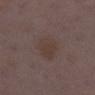image-analysis metrics: a footprint of about 9 mm² and two-axis asymmetry of about 0.2; a normalized lesion–skin contrast near 5.5; a border-irregularity index near 2/10, a color-variation rating of about 2/10, and a peripheral color-asymmetry measure near 0.5; an automated nevus-likeness rating near 0 out of 100 and lesion-presence confidence of about 100/100
body site: the right lower leg
lesion diameter: ~3.5 mm (longest diameter)
patient: female, aged 28–32
tile lighting: white-light illumination
imaging modality: ~15 mm tile from a whole-body skin photo